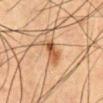workup: imaged on a skin check; not biopsied
lesion diameter: ~3.5 mm (longest diameter)
subject: male, roughly 50 years of age
lighting: cross-polarized illumination
acquisition: 15 mm crop, total-body photography
anatomic site: the abdomen